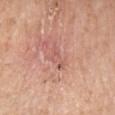notes = total-body-photography surveillance lesion; no biopsy | location = the left lower leg | patient = female, in their mid- to late 50s | image = 15 mm crop, total-body photography | diameter = ~3.5 mm (longest diameter).